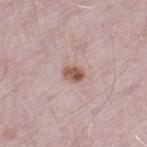Q: Is there a histopathology result?
A: total-body-photography surveillance lesion; no biopsy
Q: Who is the patient?
A: male, roughly 50 years of age
Q: Illumination type?
A: white-light
Q: What is the imaging modality?
A: ~15 mm crop, total-body skin-cancer survey
Q: Where on the body is the lesion?
A: the left thigh
Q: What is the lesion's diameter?
A: about 2.5 mm